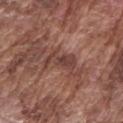  biopsy_status: not biopsied; imaged during a skin examination
  patient:
    sex: male
    age_approx: 75
  lighting: white-light
  lesion_size:
    long_diameter_mm_approx: 3.5
  image:
    source: total-body photography crop
    field_of_view_mm: 15
  automated_metrics:
    nevus_likeness_0_100: 0
    lesion_detection_confidence_0_100: 80
  site: left upper arm